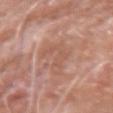The lesion was photographed on a routine skin check and not biopsied; there is no pathology result. A 15 mm crop from a total-body photograph taken for skin-cancer surveillance. A male patient, roughly 75 years of age. The lesion is located on the right forearm. This is a white-light tile. The recorded lesion diameter is about 6 mm.